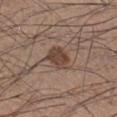{
  "biopsy_status": "not biopsied; imaged during a skin examination",
  "site": "right lower leg",
  "image": {
    "source": "total-body photography crop",
    "field_of_view_mm": 15
  },
  "patient": {
    "sex": "male",
    "age_approx": 35
  }
}